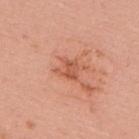Case summary:
* workup · imaged on a skin check; not biopsied